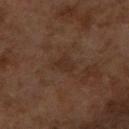Captured during whole-body skin photography for melanoma surveillance; the lesion was not biopsied. This image is a 15 mm lesion crop taken from a total-body photograph. A female subject, roughly 60 years of age. Approximately 2.5 mm at its widest. Imaged with cross-polarized lighting. The lesion is on the right forearm.